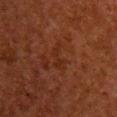Background:
This is a cross-polarized tile. A 15 mm crop from a total-body photograph taken for skin-cancer surveillance. Measured at roughly 3.5 mm in maximum diameter. From the chest. The subject is a female approximately 50 years of age.